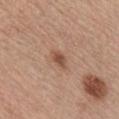  biopsy_status: not biopsied; imaged during a skin examination
  patient:
    sex: male
    age_approx: 40
  lighting: white-light
  site: chest
  lesion_size:
    long_diameter_mm_approx: 2.5
  image:
    source: total-body photography crop
    field_of_view_mm: 15
  automated_metrics:
    area_mm2_approx: 3.0
    eccentricity: 0.8
    shape_asymmetry: 0.3
    nevus_likeness_0_100: 80
    lesion_detection_confidence_0_100: 100